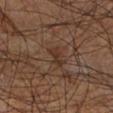Assessment:
Recorded during total-body skin imaging; not selected for excision or biopsy.
Background:
The lesion-visualizer software estimated a footprint of about 3.5 mm², an eccentricity of roughly 0.85, and a symmetry-axis asymmetry near 0.3. And it measured an average lesion color of about L≈31 a*≈17 b*≈25 (CIELAB), about 6 CIELAB-L* units darker than the surrounding skin, and a normalized lesion–skin contrast near 6.5. It also reported an automated nevus-likeness rating near 0 out of 100 and lesion-presence confidence of about 75/100. The patient is a male aged 58 to 62. Located on the leg. Captured under cross-polarized illumination. A region of skin cropped from a whole-body photographic capture, roughly 15 mm wide. The lesion's longest dimension is about 2.5 mm.